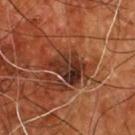Recorded during total-body skin imaging; not selected for excision or biopsy. Located on the front of the torso. The lesion-visualizer software estimated roughly 10 lightness units darker than nearby skin and a normalized border contrast of about 10.5. It also reported a border-irregularity rating of about 4/10, a within-lesion color-variation index near 7.5/10, and a peripheral color-asymmetry measure near 2.5. The analysis additionally found an automated nevus-likeness rating near 0 out of 100 and a detector confidence of about 100 out of 100 that the crop contains a lesion. A male patient approximately 55 years of age. A 15 mm close-up tile from a total-body photography series done for melanoma screening. Longest diameter approximately 5 mm. Imaged with cross-polarized lighting.